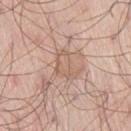follow-up = total-body-photography surveillance lesion; no biopsy.